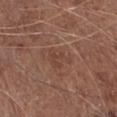Captured during whole-body skin photography for melanoma surveillance; the lesion was not biopsied.
From the right lower leg.
A male patient aged 73 to 77.
About 3.5 mm across.
Automated tile analysis of the lesion measured a footprint of about 5.5 mm² and an eccentricity of roughly 0.75. And it measured border irregularity of about 4.5 on a 0–10 scale and internal color variation of about 2 on a 0–10 scale. The software also gave a detector confidence of about 100 out of 100 that the crop contains a lesion.
A roughly 15 mm field-of-view crop from a total-body skin photograph.